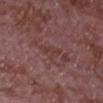Case summary:
* biopsy status: no biopsy performed (imaged during a skin exam)
* body site: the chest
* subject: male, aged approximately 65
* lighting: white-light illumination
* acquisition: total-body-photography crop, ~15 mm field of view
* lesion size: ≈3 mm